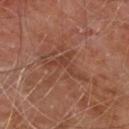Findings:
* workup — imaged on a skin check; not biopsied
* site — the chest
* TBP lesion metrics — an area of roughly 6.5 mm², a shape eccentricity near 0.75, and two-axis asymmetry of about 0.8; an average lesion color of about L≈39 a*≈22 b*≈27 (CIELAB), a lesion–skin lightness drop of about 6, and a normalized border contrast of about 5.5; a border-irregularity rating of about 10/10, a color-variation rating of about 1.5/10, and peripheral color asymmetry of about 0.5
* patient — male, roughly 60 years of age
* image — ~15 mm tile from a whole-body skin photo
* illumination — cross-polarized
* diameter — ≈5 mm